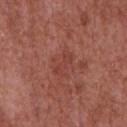notes: imaged on a skin check; not biopsied | lesion diameter: ≈3 mm | subject: male, aged 63–67 | body site: the front of the torso | imaging modality: ~15 mm tile from a whole-body skin photo | image-analysis metrics: a footprint of about 3 mm² and two-axis asymmetry of about 0.35; a lesion color around L≈42 a*≈28 b*≈27 in CIELAB and a normalized border contrast of about 4.5; a nevus-likeness score of about 0/100 and a detector confidence of about 100 out of 100 that the crop contains a lesion.